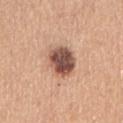  biopsy_status: not biopsied; imaged during a skin examination
  site: arm
  patient:
    sex: female
    age_approx: 65
  lighting: white-light
  image:
    source: total-body photography crop
    field_of_view_mm: 15
  automated_metrics:
    eccentricity: 0.4
    shape_asymmetry: 0.15